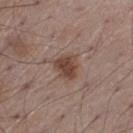The lesion was photographed on a routine skin check and not biopsied; there is no pathology result.
Captured under white-light illumination.
A male subject in their mid- to late 50s.
The lesion is on the left thigh.
The recorded lesion diameter is about 3 mm.
A 15 mm close-up tile from a total-body photography series done for melanoma screening.